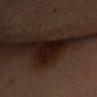<case>
  <biopsy_status>not biopsied; imaged during a skin examination</biopsy_status>
  <site>chest</site>
  <image>
    <source>total-body photography crop</source>
    <field_of_view_mm>15</field_of_view_mm>
  </image>
  <lighting>cross-polarized</lighting>
  <lesion_size>
    <long_diameter_mm_approx>7.0</long_diameter_mm_approx>
  </lesion_size>
  <patient>
    <sex>female</sex>
    <age_approx>60</age_approx>
  </patient>
</case>